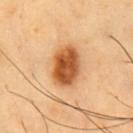Imaged during a routine full-body skin examination; the lesion was not biopsied and no histopathology is available. From the chest. A region of skin cropped from a whole-body photographic capture, roughly 15 mm wide. A male subject, roughly 60 years of age.